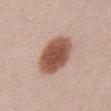{
  "image": {
    "source": "total-body photography crop",
    "field_of_view_mm": 15
  },
  "patient": {
    "sex": "female",
    "age_approx": 25
  },
  "lighting": "white-light",
  "site": "abdomen"
}